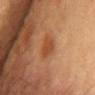Assessment:
No biopsy was performed on this lesion — it was imaged during a full skin examination and was not determined to be concerning.
Context:
Automated image analysis of the tile measured a mean CIELAB color near L≈41 a*≈22 b*≈33 and a lesion–skin lightness drop of about 7. The software also gave a color-variation rating of about 2/10 and peripheral color asymmetry of about 1. Captured under cross-polarized illumination. A male patient, aged around 85 or older. A 15 mm close-up tile from a total-body photography series done for melanoma screening. Longest diameter approximately 3 mm. The lesion is on the chest.